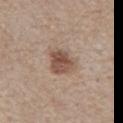  biopsy_status: not biopsied; imaged during a skin examination
  lighting: white-light
  image:
    source: total-body photography crop
    field_of_view_mm: 15
  site: chest
  lesion_size:
    long_diameter_mm_approx: 4.0
  automated_metrics:
    cielab_L: 51
    cielab_a: 17
    cielab_b: 26
    vs_skin_contrast_norm: 8.5
    nevus_likeness_0_100: 90
    lesion_detection_confidence_0_100: 100
  patient:
    sex: male
    age_approx: 80